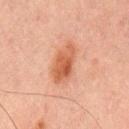Impression:
No biopsy was performed on this lesion — it was imaged during a full skin examination and was not determined to be concerning.
Image and clinical context:
A male patient in their mid-60s. The lesion is located on the mid back. A roughly 15 mm field-of-view crop from a total-body skin photograph.